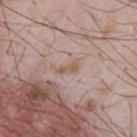Recorded during total-body skin imaging; not selected for excision or biopsy.